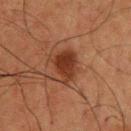biopsy_status: not biopsied; imaged during a skin examination
patient:
  sex: male
  age_approx: 60
image:
  source: total-body photography crop
  field_of_view_mm: 15
site: upper back